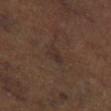Clinical impression:
No biopsy was performed on this lesion — it was imaged during a full skin examination and was not determined to be concerning.
Clinical summary:
The patient is a female in their mid- to late 60s. Cropped from a whole-body photographic skin survey; the tile spans about 15 mm. Located on the leg.